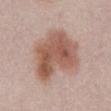{
  "lighting": "white-light",
  "image": {
    "source": "total-body photography crop",
    "field_of_view_mm": 15
  },
  "site": "abdomen",
  "lesion_size": {
    "long_diameter_mm_approx": 7.5
  },
  "patient": {
    "sex": "female",
    "age_approx": 50
  }
}